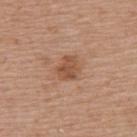Q: Was a biopsy performed?
A: no biopsy performed (imaged during a skin exam)
Q: How was this image acquired?
A: 15 mm crop, total-body photography
Q: Where on the body is the lesion?
A: the upper back
Q: Who is the patient?
A: female, in their 70s